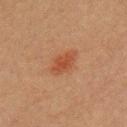Assessment:
Recorded during total-body skin imaging; not selected for excision or biopsy.
Acquisition and patient details:
The lesion is located on the chest. A male patient about 40 years old. A 15 mm close-up extracted from a 3D total-body photography capture. Measured at roughly 4 mm in maximum diameter. This is a cross-polarized tile. The total-body-photography lesion software estimated a lesion area of about 6 mm² and two-axis asymmetry of about 0.2. And it measured a mean CIELAB color near L≈40 a*≈23 b*≈30, about 7 CIELAB-L* units darker than the surrounding skin, and a normalized lesion–skin contrast near 6.5. It also reported border irregularity of about 2.5 on a 0–10 scale and a color-variation rating of about 3/10.